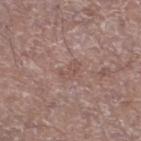This lesion was catalogued during total-body skin photography and was not selected for biopsy. A male subject aged 68 to 72. Captured under white-light illumination. Automated image analysis of the tile measured an area of roughly 3 mm², an outline eccentricity of about 0.85 (0 = round, 1 = elongated), and a shape-asymmetry score of about 0.45 (0 = symmetric). The analysis additionally found a lesion-to-skin contrast of about 5 (normalized; higher = more distinct). The analysis additionally found a border-irregularity index near 4.5/10, a within-lesion color-variation index near 0/10, and radial color variation of about 0. The analysis additionally found an automated nevus-likeness rating near 0 out of 100 and a lesion-detection confidence of about 95/100. On the left lower leg. This image is a 15 mm lesion crop taken from a total-body photograph.